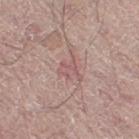Part of a total-body skin-imaging series; this lesion was reviewed on a skin check and was not flagged for biopsy.
The lesion is on the right thigh.
Longest diameter approximately 3 mm.
A male subject about 60 years old.
This image is a 15 mm lesion crop taken from a total-body photograph.
This is a white-light tile.